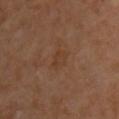Clinical impression: Recorded during total-body skin imaging; not selected for excision or biopsy. Acquisition and patient details: A male patient, about 60 years old. From the chest. Captured under cross-polarized illumination. The recorded lesion diameter is about 2.5 mm. Automated tile analysis of the lesion measured an area of roughly 4 mm², a shape eccentricity near 0.75, and two-axis asymmetry of about 0.3. And it measured an average lesion color of about L≈36 a*≈18 b*≈28 (CIELAB) and a normalized lesion–skin contrast near 4.5. A 15 mm close-up extracted from a 3D total-body photography capture.